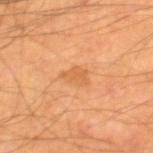* notes — total-body-photography surveillance lesion; no biopsy
* acquisition — ~15 mm crop, total-body skin-cancer survey
* lighting — cross-polarized
* location — the right thigh
* subject — male, about 65 years old